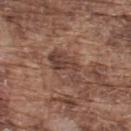| feature | finding |
|---|---|
| notes | total-body-photography surveillance lesion; no biopsy |